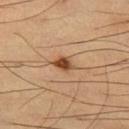Assessment: Imaged during a routine full-body skin examination; the lesion was not biopsied and no histopathology is available. Image and clinical context: A male subject, aged 58 to 62. Approximately 2.5 mm at its widest. A 15 mm close-up extracted from a 3D total-body photography capture. From the left thigh.